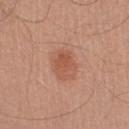| key | value |
|---|---|
| biopsy status | catalogued during a skin exam; not biopsied |
| acquisition | 15 mm crop, total-body photography |
| lesion diameter | about 4 mm |
| illumination | white-light illumination |
| TBP lesion metrics | a shape eccentricity near 0.55 and a symmetry-axis asymmetry near 0.1; an average lesion color of about L≈55 a*≈25 b*≈31 (CIELAB) and a lesion-to-skin contrast of about 6 (normalized; higher = more distinct); a classifier nevus-likeness of about 90/100 and lesion-presence confidence of about 100/100 |
| subject | male, aged 53–57 |
| location | the right lower leg |